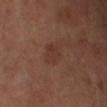Imaged during a routine full-body skin examination; the lesion was not biopsied and no histopathology is available. This image is a 15 mm lesion crop taken from a total-body photograph. A male patient, aged 63–67. Approximately 3 mm at its widest. Located on the right lower leg. Imaged with cross-polarized lighting.